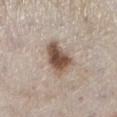Impression:
No biopsy was performed on this lesion — it was imaged during a full skin examination and was not determined to be concerning.
Context:
A male patient aged around 60. Measured at roughly 4 mm in maximum diameter. A region of skin cropped from a whole-body photographic capture, roughly 15 mm wide. Captured under white-light illumination. The lesion is located on the leg.